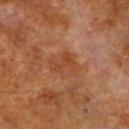Q: Was this lesion biopsied?
A: no biopsy performed (imaged during a skin exam)
Q: What lighting was used for the tile?
A: cross-polarized illumination
Q: Lesion location?
A: the front of the torso
Q: What kind of image is this?
A: ~15 mm tile from a whole-body skin photo
Q: Who is the patient?
A: male, aged approximately 80
Q: Lesion size?
A: ≈3.5 mm